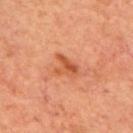– biopsy status — no biopsy performed (imaged during a skin exam)
– anatomic site — the upper back
– subject — male, approximately 70 years of age
– tile lighting — cross-polarized
– acquisition — ~15 mm crop, total-body skin-cancer survey
– lesion size — ≈3 mm